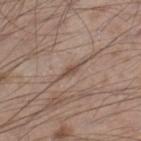No biopsy was performed on this lesion — it was imaged during a full skin examination and was not determined to be concerning.
The lesion-visualizer software estimated a lesion color around L≈49 a*≈15 b*≈25 in CIELAB and a lesion-to-skin contrast of about 6.5 (normalized; higher = more distinct). It also reported a border-irregularity rating of about 3.5/10, a color-variation rating of about 0.5/10, and peripheral color asymmetry of about 0. The analysis additionally found a classifier nevus-likeness of about 0/100.
Imaged with white-light lighting.
A close-up tile cropped from a whole-body skin photograph, about 15 mm across.
The lesion is on the right lower leg.
The lesion's longest dimension is about 3 mm.
The subject is a male aged around 35.